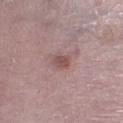Part of a total-body skin-imaging series; this lesion was reviewed on a skin check and was not flagged for biopsy. A 15 mm close-up tile from a total-body photography series done for melanoma screening. Captured under white-light illumination. The recorded lesion diameter is about 2.5 mm. A female patient aged 63 to 67. The lesion is on the left lower leg.